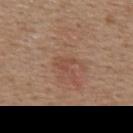The lesion was photographed on a routine skin check and not biopsied; there is no pathology result.
A 15 mm close-up tile from a total-body photography series done for melanoma screening.
The lesion-visualizer software estimated a lesion–skin lightness drop of about 6 and a lesion-to-skin contrast of about 4.5 (normalized; higher = more distinct).
From the back.
The tile uses white-light illumination.
A female subject, aged around 45.
Longest diameter approximately 3.5 mm.